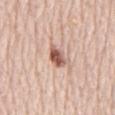Q: Was a biopsy performed?
A: total-body-photography surveillance lesion; no biopsy
Q: How was the tile lit?
A: white-light
Q: Automated lesion metrics?
A: a lesion color around L≈58 a*≈22 b*≈27 in CIELAB, roughly 16 lightness units darker than nearby skin, and a normalized lesion–skin contrast near 9.5; a border-irregularity rating of about 3/10, a within-lesion color-variation index near 5.5/10, and peripheral color asymmetry of about 2
Q: What is the anatomic site?
A: the chest
Q: What is the imaging modality?
A: ~15 mm crop, total-body skin-cancer survey
Q: What are the patient's age and sex?
A: male, aged around 80